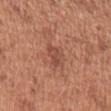Clinical impression:
The lesion was photographed on a routine skin check and not biopsied; there is no pathology result.
Image and clinical context:
An algorithmic analysis of the crop reported a lesion color around L≈48 a*≈25 b*≈29 in CIELAB, roughly 7 lightness units darker than nearby skin, and a normalized border contrast of about 5.5. And it measured a nevus-likeness score of about 0/100 and a lesion-detection confidence of about 100/100. A female subject, approximately 50 years of age. This is a white-light tile. A 15 mm close-up tile from a total-body photography series done for melanoma screening. On the left upper arm. The recorded lesion diameter is about 2.5 mm.